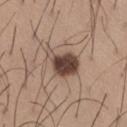Impression:
Recorded during total-body skin imaging; not selected for excision or biopsy.
Acquisition and patient details:
Cropped from a whole-body photographic skin survey; the tile spans about 15 mm. Imaged with white-light lighting. The subject is a male roughly 65 years of age. On the right thigh.